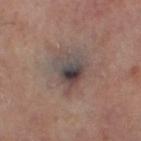The lesion is on the leg. Longest diameter approximately 5 mm. Captured under cross-polarized illumination. A 15 mm close-up tile from a total-body photography series done for melanoma screening.